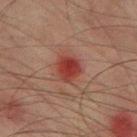workup — no biopsy performed (imaged during a skin exam).